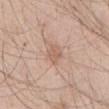Q: Was a biopsy performed?
A: no biopsy performed (imaged during a skin exam)
Q: Illumination type?
A: white-light
Q: Lesion location?
A: the front of the torso
Q: Patient demographics?
A: male, about 45 years old
Q: What did automated image analysis measure?
A: a border-irregularity rating of about 3/10, a color-variation rating of about 1.5/10, and peripheral color asymmetry of about 0.5
Q: What is the imaging modality?
A: ~15 mm tile from a whole-body skin photo